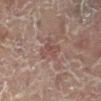biopsy status = imaged on a skin check; not biopsied
size = ~3 mm (longest diameter)
acquisition = total-body-photography crop, ~15 mm field of view
subject = female, aged 78–82
location = the leg